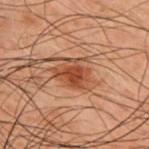Findings:
– workup · total-body-photography surveillance lesion; no biopsy
– patient · male, approximately 50 years of age
– acquisition · total-body-photography crop, ~15 mm field of view
– site · the upper back
– size · about 5 mm
– automated lesion analysis · border irregularity of about 3.5 on a 0–10 scale, a color-variation rating of about 4.5/10, and a peripheral color-asymmetry measure near 1.5